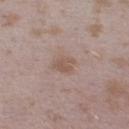follow-up = no biopsy performed (imaged during a skin exam)
automated metrics = a within-lesion color-variation index near 1.5/10 and peripheral color asymmetry of about 0.5; a classifier nevus-likeness of about 15/100 and a detector confidence of about 100 out of 100 that the crop contains a lesion
patient = female, about 25 years old
lesion diameter = ≈2.5 mm
site = the left lower leg
illumination = white-light
acquisition = ~15 mm crop, total-body skin-cancer survey